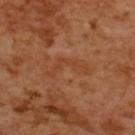Findings:
– workup · total-body-photography surveillance lesion; no biopsy
– subject · female, in their mid- to late 50s
– lesion size · ~5 mm (longest diameter)
– image source · ~15 mm crop, total-body skin-cancer survey
– site · the upper back